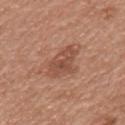The lesion was tiled from a total-body skin photograph and was not biopsied.
The recorded lesion diameter is about 4.5 mm.
Imaged with white-light lighting.
On the mid back.
A close-up tile cropped from a whole-body skin photograph, about 15 mm across.
The subject is a male roughly 65 years of age.